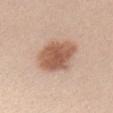A female subject approximately 30 years of age.
From the chest.
A 15 mm close-up extracted from a 3D total-body photography capture.
The total-body-photography lesion software estimated border irregularity of about 2 on a 0–10 scale, a color-variation rating of about 3.5/10, and a peripheral color-asymmetry measure near 1.